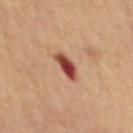workup: catalogued during a skin exam; not biopsied
lighting: cross-polarized
diameter: ~3 mm (longest diameter)
imaging modality: 15 mm crop, total-body photography
TBP lesion metrics: an average lesion color of about L≈42 a*≈26 b*≈28 (CIELAB) and a lesion–skin lightness drop of about 16; a border-irregularity index near 2.5/10 and peripheral color asymmetry of about 3.5; a nevus-likeness score of about 0/100
location: the mid back
patient: male, aged 63–67